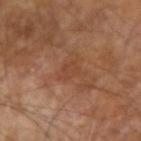This lesion was catalogued during total-body skin photography and was not selected for biopsy.
A male patient, roughly 65 years of age.
On the left arm.
Approximately 2.5 mm at its widest.
Captured under cross-polarized illumination.
A roughly 15 mm field-of-view crop from a total-body skin photograph.